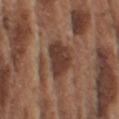lighting: white-light
image:
  source: total-body photography crop
  field_of_view_mm: 15
patient:
  sex: male
  age_approx: 75
site: arm
lesion_size:
  long_diameter_mm_approx: 4.5